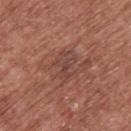This lesion was catalogued during total-body skin photography and was not selected for biopsy. This is a white-light tile. The subject is a male aged approximately 65. The total-body-photography lesion software estimated about 6 CIELAB-L* units darker than the surrounding skin and a normalized border contrast of about 5.5. The software also gave a lesion-detection confidence of about 95/100. Measured at roughly 3.5 mm in maximum diameter. The lesion is on the upper back. This image is a 15 mm lesion crop taken from a total-body photograph.